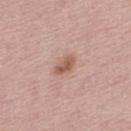follow-up = catalogued during a skin exam; not biopsied | tile lighting = white-light | TBP lesion metrics = a footprint of about 4 mm² and two-axis asymmetry of about 0.2 | subject = male, aged approximately 30 | image source = total-body-photography crop, ~15 mm field of view.